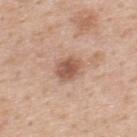biopsy status = total-body-photography surveillance lesion; no biopsy
image source = ~15 mm tile from a whole-body skin photo
tile lighting = white-light illumination
size = ≈3 mm
site = the upper back
patient = male, aged 48–52